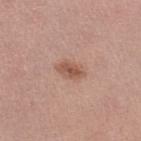Q: What kind of image is this?
A: total-body-photography crop, ~15 mm field of view
Q: How was the tile lit?
A: white-light illumination
Q: Lesion location?
A: the right lower leg
Q: Patient demographics?
A: female, roughly 35 years of age